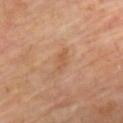Imaged during a routine full-body skin examination; the lesion was not biopsied and no histopathology is available. The subject is a male aged around 60. Cropped from a total-body skin-imaging series; the visible field is about 15 mm. The lesion-visualizer software estimated a footprint of about 3.5 mm² and a shape eccentricity near 0.9. The software also gave a mean CIELAB color near L≈54 a*≈20 b*≈34, about 6 CIELAB-L* units darker than the surrounding skin, and a normalized lesion–skin contrast near 5. The analysis additionally found a border-irregularity index near 4/10 and internal color variation of about 1 on a 0–10 scale. And it measured lesion-presence confidence of about 100/100. The lesion is on the chest. The tile uses cross-polarized illumination.